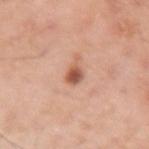The lesion was photographed on a routine skin check and not biopsied; there is no pathology result. Located on the left upper arm. A male patient approximately 55 years of age. Automated image analysis of the tile measured a shape-asymmetry score of about 0.25 (0 = symmetric). It also reported an automated nevus-likeness rating near 95 out of 100 and a detector confidence of about 100 out of 100 that the crop contains a lesion. A close-up tile cropped from a whole-body skin photograph, about 15 mm across. The tile uses white-light illumination.